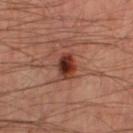Part of a total-body skin-imaging series; this lesion was reviewed on a skin check and was not flagged for biopsy.
The lesion is on the right thigh.
A close-up tile cropped from a whole-body skin photograph, about 15 mm across.
The patient is a male in their mid- to late 50s.
Captured under cross-polarized illumination.
Measured at roughly 3 mm in maximum diameter.